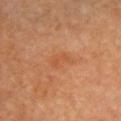The lesion was photographed on a routine skin check and not biopsied; there is no pathology result. The lesion's longest dimension is about 3 mm. The subject is a female aged 63–67. A 15 mm crop from a total-body photograph taken for skin-cancer surveillance. On the chest.